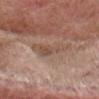Impression: The lesion was tiled from a total-body skin photograph and was not biopsied. Acquisition and patient details: The lesion is located on the head or neck. This image is a 15 mm lesion crop taken from a total-body photograph. The tile uses white-light illumination. About 5.5 mm across. A male patient roughly 75 years of age.